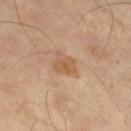No biopsy was performed on this lesion — it was imaged during a full skin examination and was not determined to be concerning. The lesion is on the right thigh. About 2.5 mm across. A male patient aged approximately 65. A 15 mm close-up tile from a total-body photography series done for melanoma screening. Imaged with cross-polarized lighting.